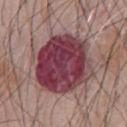The lesion was photographed on a routine skin check and not biopsied; there is no pathology result.
The patient is a male approximately 60 years of age.
Located on the abdomen.
Cropped from a whole-body photographic skin survey; the tile spans about 15 mm.
Approximately 8 mm at its widest.